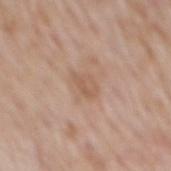Impression:
Part of a total-body skin-imaging series; this lesion was reviewed on a skin check and was not flagged for biopsy.
Image and clinical context:
A male subject, aged 63 to 67. Located on the mid back. This is a white-light tile. A 15 mm crop from a total-body photograph taken for skin-cancer surveillance. Approximately 3 mm at its widest.